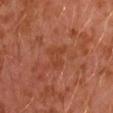Imaged during a routine full-body skin examination; the lesion was not biopsied and no histopathology is available.
Longest diameter approximately 3 mm.
An algorithmic analysis of the crop reported a footprint of about 5.5 mm², an eccentricity of roughly 0.65, and two-axis asymmetry of about 0.45. And it measured a mean CIELAB color near L≈42 a*≈27 b*≈33, roughly 5 lightness units darker than nearby skin, and a lesion-to-skin contrast of about 4.5 (normalized; higher = more distinct).
The tile uses cross-polarized illumination.
The patient is a male aged around 30.
A roughly 15 mm field-of-view crop from a total-body skin photograph.
Located on the left arm.